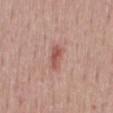Captured during whole-body skin photography for melanoma surveillance; the lesion was not biopsied. The tile uses white-light illumination. From the mid back. A 15 mm close-up tile from a total-body photography series done for melanoma screening. The lesion's longest dimension is about 3 mm. The patient is a male roughly 50 years of age.